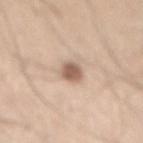| field | value |
|---|---|
| follow-up | no biopsy performed (imaged during a skin exam) |
| image source | ~15 mm tile from a whole-body skin photo |
| site | the mid back |
| lesion diameter | ~3 mm (longest diameter) |
| patient | male, aged around 45 |